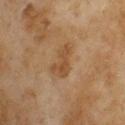  biopsy_status: not biopsied; imaged during a skin examination
  image:
    source: total-body photography crop
    field_of_view_mm: 15
  site: upper back
  patient:
    sex: female
    age_approx: 60
  lesion_size:
    long_diameter_mm_approx: 4.0
  automated_metrics:
    area_mm2_approx: 7.0
    eccentricity: 0.8
    cielab_L: 44
    cielab_a: 17
    cielab_b: 33
    vs_skin_darker_L: 7.0
    vs_skin_contrast_norm: 6.0
    border_irregularity_0_10: 4.0
    color_variation_0_10: 2.5
    peripheral_color_asymmetry: 1.0